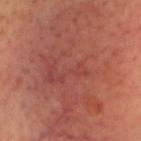{
  "biopsy_status": "not biopsied; imaged during a skin examination",
  "lighting": "cross-polarized",
  "site": "head or neck",
  "patient": {
    "sex": "male",
    "age_approx": 45
  },
  "image": {
    "source": "total-body photography crop",
    "field_of_view_mm": 15
  },
  "automated_metrics": {
    "border_irregularity_0_10": 7.0,
    "color_variation_0_10": 5.5,
    "peripheral_color_asymmetry": 2.0
  },
  "lesion_size": {
    "long_diameter_mm_approx": 16.0
  }
}